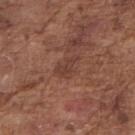Impression:
Imaged during a routine full-body skin examination; the lesion was not biopsied and no histopathology is available.
Context:
The lesion's longest dimension is about 3 mm. Imaged with white-light lighting. A male subject, aged around 75. An algorithmic analysis of the crop reported a mean CIELAB color near L≈40 a*≈22 b*≈26, about 6 CIELAB-L* units darker than the surrounding skin, and a normalized border contrast of about 5.5. The software also gave a border-irregularity index near 4.5/10, internal color variation of about 2 on a 0–10 scale, and a peripheral color-asymmetry measure near 0.5. The software also gave a nevus-likeness score of about 0/100 and a lesion-detection confidence of about 100/100. Located on the right upper arm. A 15 mm close-up extracted from a 3D total-body photography capture.